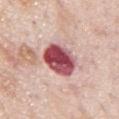No biopsy was performed on this lesion — it was imaged during a full skin examination and was not determined to be concerning.